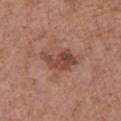• notes — catalogued during a skin exam; not biopsied
• automated lesion analysis — a lesion color around L≈46 a*≈22 b*≈27 in CIELAB, a lesion–skin lightness drop of about 10, and a lesion-to-skin contrast of about 8 (normalized; higher = more distinct); border irregularity of about 3.5 on a 0–10 scale, a within-lesion color-variation index near 4.5/10, and a peripheral color-asymmetry measure near 1.5
• lesion size — ≈4.5 mm
• anatomic site — the arm
• lighting — white-light illumination
• acquisition — ~15 mm tile from a whole-body skin photo
• patient — female, aged approximately 75